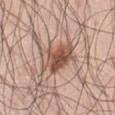{"patient": {"sex": "male", "age_approx": 70}, "image": {"source": "total-body photography crop", "field_of_view_mm": 15}, "lesion_size": {"long_diameter_mm_approx": 5.0}, "automated_metrics": {"shape_asymmetry": 0.25, "cielab_L": 53, "cielab_a": 20, "cielab_b": 27, "vs_skin_darker_L": 13.0, "vs_skin_contrast_norm": 8.5, "color_variation_0_10": 8.5, "peripheral_color_asymmetry": 3.0, "nevus_likeness_0_100": 90}, "site": "front of the torso", "lighting": "white-light"}